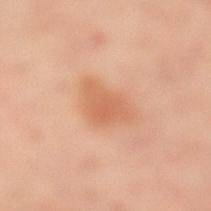Q: Was a biopsy performed?
A: no biopsy performed (imaged during a skin exam)
Q: What kind of image is this?
A: 15 mm crop, total-body photography
Q: What are the patient's age and sex?
A: female, aged 38 to 42
Q: Where on the body is the lesion?
A: the right leg